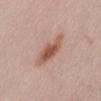notes — imaged on a skin check; not biopsied | image source — ~15 mm crop, total-body skin-cancer survey | lesion diameter — about 4.5 mm | patient — male, about 55 years old | body site — the abdomen | TBP lesion metrics — a footprint of about 8.5 mm², a shape eccentricity near 0.85, and a symmetry-axis asymmetry near 0.25; a mean CIELAB color near L≈55 a*≈22 b*≈28 and a lesion-to-skin contrast of about 8.5 (normalized; higher = more distinct); a border-irregularity index near 2.5/10 and a within-lesion color-variation index near 4/10; a nevus-likeness score of about 90/100 | lighting — white-light.